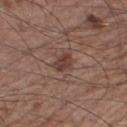notes — total-body-photography surveillance lesion; no biopsy | acquisition — ~15 mm crop, total-body skin-cancer survey | subject — male, in their 60s | site — the right thigh.